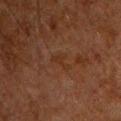| field | value |
|---|---|
| size | ~2.5 mm (longest diameter) |
| patient | male, aged 58–62 |
| illumination | cross-polarized illumination |
| location | the front of the torso |
| image source | total-body-photography crop, ~15 mm field of view |